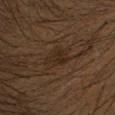Background: The lesion is located on the head or neck. This image is a 15 mm lesion crop taken from a total-body photograph. The patient is a male aged around 55.